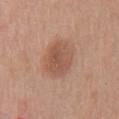This is a white-light tile. A male patient, aged 58 to 62. The lesion-visualizer software estimated an average lesion color of about L≈54 a*≈21 b*≈29 (CIELAB) and a lesion-to-skin contrast of about 6.5 (normalized; higher = more distinct). The analysis additionally found an automated nevus-likeness rating near 65 out of 100 and a detector confidence of about 100 out of 100 that the crop contains a lesion. Cropped from a whole-body photographic skin survey; the tile spans about 15 mm. On the chest. Approximately 4.5 mm at its widest.